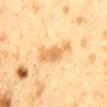A close-up tile cropped from a whole-body skin photograph, about 15 mm across.
Imaged with cross-polarized lighting.
The lesion-visualizer software estimated a lesion color around L≈59 a*≈17 b*≈39 in CIELAB and a lesion–skin lightness drop of about 10. It also reported border irregularity of about 4.5 on a 0–10 scale, a color-variation rating of about 2/10, and a peripheral color-asymmetry measure near 0.5. It also reported lesion-presence confidence of about 100/100.
A female patient, in their 40s.
Approximately 5.5 mm at its widest.
The lesion is located on the mid back.